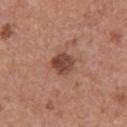No biopsy was performed on this lesion — it was imaged during a full skin examination and was not determined to be concerning. A male patient aged approximately 45. The tile uses white-light illumination. The lesion is located on the left upper arm. A region of skin cropped from a whole-body photographic capture, roughly 15 mm wide.